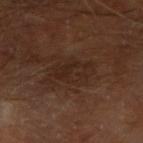Recorded during total-body skin imaging; not selected for excision or biopsy. An algorithmic analysis of the crop reported a border-irregularity rating of about 4/10, a color-variation rating of about 2.5/10, and radial color variation of about 1. The analysis additionally found a detector confidence of about 95 out of 100 that the crop contains a lesion. A male subject, in their mid-60s. From the left forearm. This is a cross-polarized tile. Longest diameter approximately 4.5 mm. A lesion tile, about 15 mm wide, cut from a 3D total-body photograph.